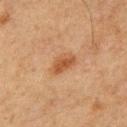follow-up: catalogued during a skin exam; not biopsied | subject: male, in their 50s | lesion size: ≈3.5 mm | lighting: cross-polarized | location: the chest | automated lesion analysis: a lesion area of about 6 mm², a shape eccentricity near 0.8, and a shape-asymmetry score of about 0.2 (0 = symmetric) | imaging modality: 15 mm crop, total-body photography.